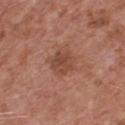Impression: Part of a total-body skin-imaging series; this lesion was reviewed on a skin check and was not flagged for biopsy. Background: The lesion is on the chest. A close-up tile cropped from a whole-body skin photograph, about 15 mm across. Automated tile analysis of the lesion measured a footprint of about 7 mm², an outline eccentricity of about 0.3 (0 = round, 1 = elongated), and two-axis asymmetry of about 0.2. The analysis additionally found an average lesion color of about L≈46 a*≈23 b*≈29 (CIELAB), a lesion–skin lightness drop of about 8, and a lesion-to-skin contrast of about 6.5 (normalized; higher = more distinct). The analysis additionally found a classifier nevus-likeness of about 15/100 and a detector confidence of about 100 out of 100 that the crop contains a lesion. A male patient, aged 73 to 77. About 3 mm across. The tile uses white-light illumination.